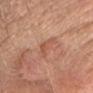| field | value |
|---|---|
| notes | no biopsy performed (imaged during a skin exam) |
| image | total-body-photography crop, ~15 mm field of view |
| lesion diameter | about 4 mm |
| illumination | white-light |
| automated metrics | an eccentricity of roughly 0.95 and two-axis asymmetry of about 0.6; a lesion color around L≈55 a*≈25 b*≈32 in CIELAB; a classifier nevus-likeness of about 5/100 and lesion-presence confidence of about 100/100 |
| body site | the head or neck |
| subject | female, aged 53 to 57 |